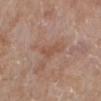Findings:
– workup: total-body-photography surveillance lesion; no biopsy
– tile lighting: white-light
– body site: the right lower leg
– automated metrics: an automated nevus-likeness rating near 0 out of 100
– imaging modality: ~15 mm crop, total-body skin-cancer survey
– subject: female, aged approximately 75
– lesion diameter: about 3 mm